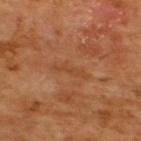workup: total-body-photography surveillance lesion; no biopsy | imaging modality: ~15 mm crop, total-body skin-cancer survey | diameter: ~4 mm (longest diameter) | anatomic site: the upper back | subject: male, approximately 65 years of age | automated metrics: a mean CIELAB color near L≈44 a*≈24 b*≈36, about 5 CIELAB-L* units darker than the surrounding skin, and a normalized border contrast of about 4.5; a nevus-likeness score of about 0/100 | lighting: cross-polarized.